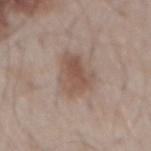– workup — catalogued during a skin exam; not biopsied
– imaging modality — total-body-photography crop, ~15 mm field of view
– automated lesion analysis — a lesion area of about 12 mm², an outline eccentricity of about 0.65 (0 = round, 1 = elongated), and a shape-asymmetry score of about 0.35 (0 = symmetric); an average lesion color of about L≈52 a*≈17 b*≈24 (CIELAB), about 9 CIELAB-L* units darker than the surrounding skin, and a lesion-to-skin contrast of about 7 (normalized; higher = more distinct); border irregularity of about 3.5 on a 0–10 scale; lesion-presence confidence of about 100/100
– location — the back
– patient — male, aged 53–57
– diameter — ≈5 mm